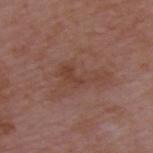Case summary:
– workup — no biopsy performed (imaged during a skin exam)
– patient — male, about 50 years old
– image-analysis metrics — an area of roughly 6.5 mm², an eccentricity of roughly 0.9, and two-axis asymmetry of about 0.6; a color-variation rating of about 1.5/10
– acquisition — ~15 mm crop, total-body skin-cancer survey
– size — ≈5.5 mm
– lighting — white-light
– location — the upper back